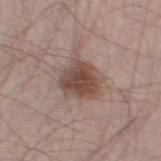Part of a total-body skin-imaging series; this lesion was reviewed on a skin check and was not flagged for biopsy. The lesion is located on the left thigh. The patient is a male aged 53–57. Measured at roughly 4.5 mm in maximum diameter. Captured under white-light illumination. This image is a 15 mm lesion crop taken from a total-body photograph.